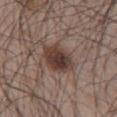subject = male, aged around 65 | diameter = ~5 mm (longest diameter) | site = the front of the torso | imaging modality = total-body-photography crop, ~15 mm field of view | illumination = white-light.